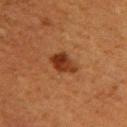The lesion was photographed on a routine skin check and not biopsied; there is no pathology result. The tile uses cross-polarized illumination. Approximately 3.5 mm at its widest. This image is a 15 mm lesion crop taken from a total-body photograph. From the upper back. A female subject, aged approximately 40. Automated tile analysis of the lesion measured a border-irregularity index near 2.5/10, a within-lesion color-variation index near 5/10, and radial color variation of about 2. The software also gave an automated nevus-likeness rating near 100 out of 100 and lesion-presence confidence of about 100/100.